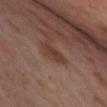Clinical impression:
This lesion was catalogued during total-body skin photography and was not selected for biopsy.
Acquisition and patient details:
A female patient, in their mid- to late 50s. The tile uses cross-polarized illumination. From the right thigh. Approximately 3 mm at its widest. Cropped from a total-body skin-imaging series; the visible field is about 15 mm.